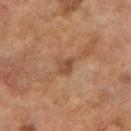diameter: ~2.5 mm (longest diameter)
patient: female, roughly 60 years of age
acquisition: ~15 mm crop, total-body skin-cancer survey
site: the right forearm
lighting: cross-polarized illumination